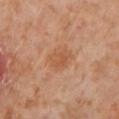Impression:
The lesion was photographed on a routine skin check and not biopsied; there is no pathology result.
Background:
The subject is a female aged 53–57. A 15 mm close-up extracted from a 3D total-body photography capture. On the left lower leg.